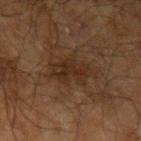Case summary:
- notes — total-body-photography surveillance lesion; no biopsy
- lighting — cross-polarized illumination
- lesion diameter — ≈5 mm
- body site — the left upper arm
- subject — male, aged 68–72
- imaging modality — 15 mm crop, total-body photography